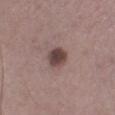imaging modality = total-body-photography crop, ~15 mm field of view
anatomic site = the leg
tile lighting = white-light illumination
subject = male, in their mid-50s
automated metrics = a nevus-likeness score of about 65/100
size = ~2.5 mm (longest diameter)